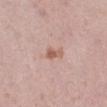notes=no biopsy performed (imaged during a skin exam)
patient=female, aged 38–42
anatomic site=the left lower leg
image source=~15 mm tile from a whole-body skin photo